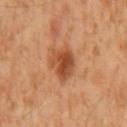Captured during whole-body skin photography for melanoma surveillance; the lesion was not biopsied. A region of skin cropped from a whole-body photographic capture, roughly 15 mm wide. Measured at roughly 3.5 mm in maximum diameter. On the mid back. A male patient, aged approximately 60. Imaged with cross-polarized lighting. An algorithmic analysis of the crop reported a footprint of about 8.5 mm² and an outline eccentricity of about 0.4 (0 = round, 1 = elongated).